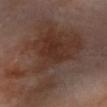Findings:
- biopsy status: imaged on a skin check; not biopsied
- patient: male, aged 53 to 57
- lesion size: ≈13.5 mm
- lighting: cross-polarized
- site: the left forearm
- automated lesion analysis: an area of roughly 55 mm², an outline eccentricity of about 0.85 (0 = round, 1 = elongated), and a symmetry-axis asymmetry near 0.55; an average lesion color of about L≈35 a*≈17 b*≈23 (CIELAB), a lesion–skin lightness drop of about 9, and a normalized border contrast of about 8.5; a border-irregularity rating of about 8.5/10 and peripheral color asymmetry of about 1; a nevus-likeness score of about 10/100 and lesion-presence confidence of about 95/100
- image: total-body-photography crop, ~15 mm field of view